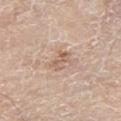Findings:
- notes — imaged on a skin check; not biopsied
- subject — male, roughly 80 years of age
- image source — ~15 mm crop, total-body skin-cancer survey
- size — ~3 mm (longest diameter)
- body site — the left thigh